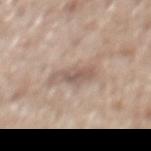biopsy status: imaged on a skin check; not biopsied
lesion diameter: ~4 mm (longest diameter)
body site: the mid back
automated metrics: a lesion area of about 6 mm², an outline eccentricity of about 0.85 (0 = round, 1 = elongated), and a shape-asymmetry score of about 0.4 (0 = symmetric); a lesion color around L≈57 a*≈15 b*≈24 in CIELAB, a lesion–skin lightness drop of about 10, and a lesion-to-skin contrast of about 6.5 (normalized; higher = more distinct); a border-irregularity rating of about 5/10, internal color variation of about 2.5 on a 0–10 scale, and a peripheral color-asymmetry measure near 0.5
imaging modality: total-body-photography crop, ~15 mm field of view
subject: male, aged approximately 65
tile lighting: white-light